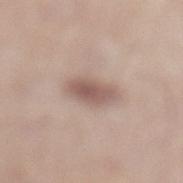Recorded during total-body skin imaging; not selected for excision or biopsy. A male patient, in their mid- to late 30s. Approximately 4 mm at its widest. Cropped from a total-body skin-imaging series; the visible field is about 15 mm. From the leg.